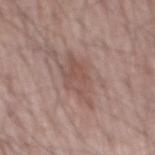Clinical impression: Recorded during total-body skin imaging; not selected for excision or biopsy. Background: Located on the mid back. The lesion's longest dimension is about 6.5 mm. A 15 mm close-up extracted from a 3D total-body photography capture. Automated image analysis of the tile measured an average lesion color of about L≈52 a*≈19 b*≈23 (CIELAB) and a normalized border contrast of about 6. It also reported border irregularity of about 5 on a 0–10 scale, internal color variation of about 3 on a 0–10 scale, and radial color variation of about 1. The software also gave a nevus-likeness score of about 0/100 and a lesion-detection confidence of about 100/100. A male patient approximately 65 years of age.